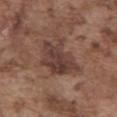This lesion was catalogued during total-body skin photography and was not selected for biopsy.
A roughly 15 mm field-of-view crop from a total-body skin photograph.
A male patient, about 75 years old.
This is a white-light tile.
The lesion is on the abdomen.
The lesion's longest dimension is about 5.5 mm.